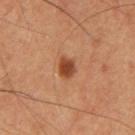Captured during whole-body skin photography for melanoma surveillance; the lesion was not biopsied. A roughly 15 mm field-of-view crop from a total-body skin photograph. Longest diameter approximately 2.5 mm. The subject is a male aged 58 to 62. Located on the leg. Captured under cross-polarized illumination. Automated tile analysis of the lesion measured a footprint of about 4.5 mm², a shape eccentricity near 0.55, and a shape-asymmetry score of about 0.25 (0 = symmetric). And it measured an average lesion color of about L≈41 a*≈24 b*≈32 (CIELAB), about 12 CIELAB-L* units darker than the surrounding skin, and a normalized border contrast of about 9.5.